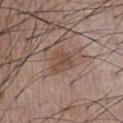| key | value |
|---|---|
| follow-up | total-body-photography surveillance lesion; no biopsy |
| patient | male, about 55 years old |
| size | ≈3.5 mm |
| image source | ~15 mm crop, total-body skin-cancer survey |
| illumination | white-light |
| image-analysis metrics | border irregularity of about 3.5 on a 0–10 scale, a color-variation rating of about 3/10, and a peripheral color-asymmetry measure near 1; an automated nevus-likeness rating near 15 out of 100 and a lesion-detection confidence of about 100/100 |
| site | the chest |